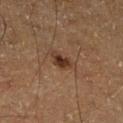  biopsy_status: not biopsied; imaged during a skin examination
  patient:
    sex: male
    age_approx: 65
  image:
    source: total-body photography crop
    field_of_view_mm: 15
  lighting: cross-polarized
  lesion_size:
    long_diameter_mm_approx: 3.0
  site: leg